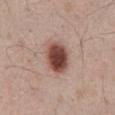Clinical summary:
The patient is a male aged around 65. Automated image analysis of the tile measured a color-variation rating of about 5.5/10 and a peripheral color-asymmetry measure near 1.5. A 15 mm close-up tile from a total-body photography series done for melanoma screening. The lesion is located on the abdomen. The recorded lesion diameter is about 4 mm.